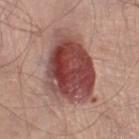Q: Was a biopsy performed?
A: catalogued during a skin exam; not biopsied
Q: What kind of image is this?
A: ~15 mm crop, total-body skin-cancer survey
Q: Patient demographics?
A: male, roughly 50 years of age
Q: How large is the lesion?
A: ≈8.5 mm
Q: Lesion location?
A: the right lower leg
Q: What did automated image analysis measure?
A: a lesion area of about 36 mm², an outline eccentricity of about 0.8 (0 = round, 1 = elongated), and a shape-asymmetry score of about 0.25 (0 = symmetric); an average lesion color of about L≈46 a*≈25 b*≈23 (CIELAB), about 17 CIELAB-L* units darker than the surrounding skin, and a lesion-to-skin contrast of about 12 (normalized; higher = more distinct); a nevus-likeness score of about 95/100 and lesion-presence confidence of about 100/100